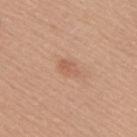The lesion was photographed on a routine skin check and not biopsied; there is no pathology result. The subject is a female in their mid- to late 50s. Located on the upper back. The lesion's longest dimension is about 3 mm. A 15 mm close-up extracted from a 3D total-body photography capture. An algorithmic analysis of the crop reported roughly 7 lightness units darker than nearby skin and a lesion-to-skin contrast of about 5 (normalized; higher = more distinct).